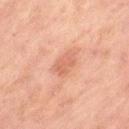Q: Was a biopsy performed?
A: imaged on a skin check; not biopsied
Q: What is the lesion's diameter?
A: ~3 mm (longest diameter)
Q: Lesion location?
A: the left thigh
Q: Automated lesion metrics?
A: an area of roughly 5.5 mm², an eccentricity of roughly 0.75, and two-axis asymmetry of about 0.25; an average lesion color of about L≈56 a*≈24 b*≈30 (CIELAB) and roughly 8 lightness units darker than nearby skin; an automated nevus-likeness rating near 0 out of 100 and a lesion-detection confidence of about 100/100
Q: What are the patient's age and sex?
A: female, about 60 years old
Q: What kind of image is this?
A: 15 mm crop, total-body photography
Q: What lighting was used for the tile?
A: cross-polarized